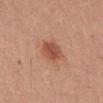Q: Lesion location?
A: the mid back
Q: Illumination type?
A: white-light illumination
Q: What kind of image is this?
A: ~15 mm crop, total-body skin-cancer survey
Q: Lesion size?
A: ≈3 mm
Q: Who is the patient?
A: male, in their mid- to late 40s